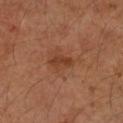follow-up=no biopsy performed (imaged during a skin exam)
body site=the left forearm
imaging modality=~15 mm crop, total-body skin-cancer survey
image-analysis metrics=a lesion area of about 3.5 mm², an eccentricity of roughly 0.9, and a symmetry-axis asymmetry near 0.3; a lesion color around L≈40 a*≈25 b*≈34 in CIELAB and roughly 8 lightness units darker than nearby skin; border irregularity of about 3 on a 0–10 scale, a within-lesion color-variation index near 1/10, and a peripheral color-asymmetry measure near 0
diameter=about 2.5 mm
subject=female, in their 60s